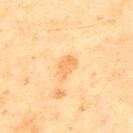A male patient in their mid-40s. The lesion is located on the upper back. Automated tile analysis of the lesion measured an area of roughly 4.5 mm² and an eccentricity of roughly 0.8. The analysis additionally found a within-lesion color-variation index near 2.5/10 and radial color variation of about 1. A close-up tile cropped from a whole-body skin photograph, about 15 mm across. The recorded lesion diameter is about 3 mm. The tile uses cross-polarized illumination.